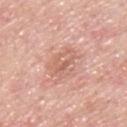follow-up — catalogued during a skin exam; not biopsied
body site — the back
acquisition — total-body-photography crop, ~15 mm field of view
subject — male, aged 43 to 47
automated metrics — a footprint of about 9.5 mm², an eccentricity of roughly 0.85, and a symmetry-axis asymmetry near 0.2; border irregularity of about 2.5 on a 0–10 scale, a color-variation rating of about 5/10, and a peripheral color-asymmetry measure near 1.5; a nevus-likeness score of about 0/100